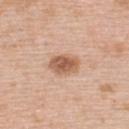Q: Was this lesion biopsied?
A: imaged on a skin check; not biopsied
Q: What is the lesion's diameter?
A: ~3.5 mm (longest diameter)
Q: What are the patient's age and sex?
A: male, aged 58–62
Q: What kind of image is this?
A: total-body-photography crop, ~15 mm field of view
Q: Where on the body is the lesion?
A: the back
Q: What lighting was used for the tile?
A: white-light illumination